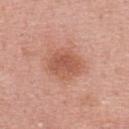Q: Was this lesion biopsied?
A: imaged on a skin check; not biopsied
Q: Lesion location?
A: the upper back
Q: What is the imaging modality?
A: ~15 mm tile from a whole-body skin photo
Q: Who is the patient?
A: female, aged around 40
Q: How was the tile lit?
A: white-light
Q: How large is the lesion?
A: ≈4 mm
Q: Automated lesion metrics?
A: a border-irregularity rating of about 2/10 and radial color variation of about 1; a classifier nevus-likeness of about 35/100 and lesion-presence confidence of about 100/100